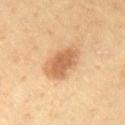Impression:
Part of a total-body skin-imaging series; this lesion was reviewed on a skin check and was not flagged for biopsy.
Background:
Located on the chest. A 15 mm crop from a total-body photograph taken for skin-cancer surveillance. This is a cross-polarized tile. A male subject, aged approximately 60. Automated image analysis of the tile measured an eccentricity of roughly 0.75. The software also gave border irregularity of about 2 on a 0–10 scale and a within-lesion color-variation index near 3.5/10. About 5 mm across.